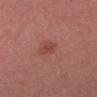No biopsy was performed on this lesion — it was imaged during a full skin examination and was not determined to be concerning.
This is a white-light tile.
A female patient aged around 25.
Automated image analysis of the tile measured an average lesion color of about L≈45 a*≈26 b*≈26 (CIELAB), a lesion–skin lightness drop of about 7, and a normalized border contrast of about 6. It also reported internal color variation of about 2 on a 0–10 scale and radial color variation of about 0.5. The software also gave a nevus-likeness score of about 60/100.
A close-up tile cropped from a whole-body skin photograph, about 15 mm across.
On the head or neck.
Measured at roughly 2.5 mm in maximum diameter.